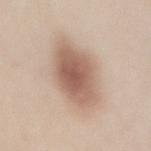This lesion was catalogued during total-body skin photography and was not selected for biopsy. The tile uses white-light illumination. A female patient aged approximately 30. Approximately 6.5 mm at its widest. Automated tile analysis of the lesion measured a mean CIELAB color near L≈60 a*≈18 b*≈27, about 13 CIELAB-L* units darker than the surrounding skin, and a lesion-to-skin contrast of about 8.5 (normalized; higher = more distinct). And it measured a classifier nevus-likeness of about 100/100 and lesion-presence confidence of about 100/100. A 15 mm crop from a total-body photograph taken for skin-cancer surveillance. The lesion is on the mid back.